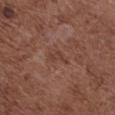Longest diameter approximately 2.5 mm. A female subject, roughly 80 years of age. Cropped from a whole-body photographic skin survey; the tile spans about 15 mm. From the chest. This is a white-light tile.